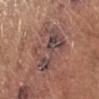The lesion was photographed on a routine skin check and not biopsied; there is no pathology result.
The recorded lesion diameter is about 6 mm.
The tile uses white-light illumination.
A roughly 15 mm field-of-view crop from a total-body skin photograph.
A male subject aged approximately 70.
The total-body-photography lesion software estimated a lesion color around L≈45 a*≈18 b*≈20 in CIELAB, roughly 8 lightness units darker than nearby skin, and a normalized border contrast of about 8. The software also gave border irregularity of about 7 on a 0–10 scale, internal color variation of about 9 on a 0–10 scale, and radial color variation of about 3.5.
The lesion is located on the arm.